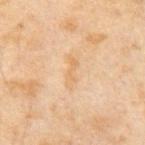Clinical impression: Part of a total-body skin-imaging series; this lesion was reviewed on a skin check and was not flagged for biopsy. Clinical summary: A 15 mm crop from a total-body photograph taken for skin-cancer surveillance. The tile uses cross-polarized illumination. A male subject in their mid- to late 60s.